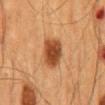Q: Is there a histopathology result?
A: no biopsy performed (imaged during a skin exam)
Q: Where on the body is the lesion?
A: the mid back
Q: What is the lesion's diameter?
A: ~4 mm (longest diameter)
Q: What is the imaging modality?
A: total-body-photography crop, ~15 mm field of view
Q: What are the patient's age and sex?
A: male, aged approximately 65